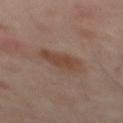Impression:
Part of a total-body skin-imaging series; this lesion was reviewed on a skin check and was not flagged for biopsy.
Acquisition and patient details:
On the mid back. A 15 mm crop from a total-body photograph taken for skin-cancer surveillance. A patient aged 53–57. About 4 mm across.